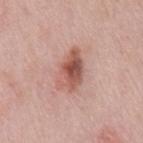biopsy_status: not biopsied; imaged during a skin examination
site: front of the torso
lesion_size:
  long_diameter_mm_approx: 5.0
image:
  source: total-body photography crop
  field_of_view_mm: 15
patient:
  sex: male
  age_approx: 50